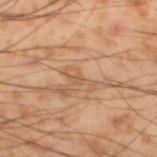Assessment: The lesion was tiled from a total-body skin photograph and was not biopsied. Acquisition and patient details: The subject is a male aged approximately 55. A lesion tile, about 15 mm wide, cut from a 3D total-body photograph. Imaged with cross-polarized lighting. Located on the left lower leg. Automated image analysis of the tile measured a shape eccentricity near 0.95 and a symmetry-axis asymmetry near 0.5. And it measured a mean CIELAB color near L≈58 a*≈20 b*≈36, roughly 7 lightness units darker than nearby skin, and a normalized border contrast of about 5. And it measured a border-irregularity rating of about 6/10, internal color variation of about 2 on a 0–10 scale, and radial color variation of about 0.5.